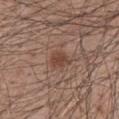Notes:
– diameter — ≈2.5 mm
– illumination — white-light
– site — the mid back
– acquisition — total-body-photography crop, ~15 mm field of view
– patient — male, in their 50s